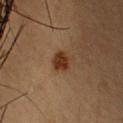Q: Was this lesion biopsied?
A: no biopsy performed (imaged during a skin exam)
Q: How was the tile lit?
A: cross-polarized
Q: What is the imaging modality?
A: total-body-photography crop, ~15 mm field of view
Q: Who is the patient?
A: female, about 45 years old
Q: Automated lesion metrics?
A: a lesion area of about 5 mm², an eccentricity of roughly 0.35, and a symmetry-axis asymmetry near 0.15; a normalized lesion–skin contrast near 10; an automated nevus-likeness rating near 100 out of 100 and a lesion-detection confidence of about 100/100
Q: What is the anatomic site?
A: the upper back